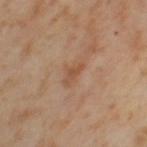Clinical impression:
The lesion was tiled from a total-body skin photograph and was not biopsied.
Image and clinical context:
A roughly 15 mm field-of-view crop from a total-body skin photograph. Imaged with cross-polarized lighting. The subject is a female in their mid- to late 50s. Automated image analysis of the tile measured a mean CIELAB color near L≈51 a*≈21 b*≈33 and roughly 8 lightness units darker than nearby skin. And it measured a border-irregularity rating of about 5.5/10, a within-lesion color-variation index near 0/10, and radial color variation of about 0. The software also gave a lesion-detection confidence of about 100/100. Longest diameter approximately 2.5 mm. From the leg.